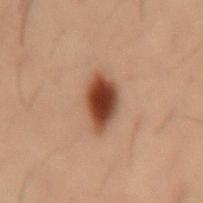The lesion was photographed on a routine skin check and not biopsied; there is no pathology result. About 5 mm across. This is a cross-polarized tile. A 15 mm close-up extracted from a 3D total-body photography capture. The lesion-visualizer software estimated internal color variation of about 4.5 on a 0–10 scale and peripheral color asymmetry of about 1. The analysis additionally found an automated nevus-likeness rating near 100 out of 100. The lesion is on the right thigh. The patient is a male roughly 30 years of age.